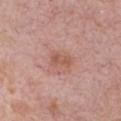- tile lighting — white-light illumination
- acquisition — 15 mm crop, total-body photography
- body site — the left upper arm
- patient — male, approximately 75 years of age
- lesion size — about 3 mm